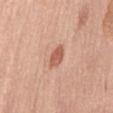This lesion was catalogued during total-body skin photography and was not selected for biopsy.